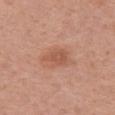follow-up: imaged on a skin check; not biopsied | patient: female, aged 33–37 | size: about 3.5 mm | body site: the chest | automated lesion analysis: a border-irregularity rating of about 4/10, internal color variation of about 1.5 on a 0–10 scale, and peripheral color asymmetry of about 0.5; an automated nevus-likeness rating near 45 out of 100 | image source: 15 mm crop, total-body photography | illumination: white-light illumination.